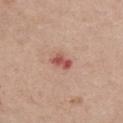| field | value |
|---|---|
| follow-up | no biopsy performed (imaged during a skin exam) |
| automated lesion analysis | an area of roughly 3.5 mm², an eccentricity of roughly 0.8, and a shape-asymmetry score of about 0.3 (0 = symmetric); an average lesion color of about L≈54 a*≈29 b*≈26 (CIELAB), roughly 12 lightness units darker than nearby skin, and a normalized lesion–skin contrast near 8.5; a border-irregularity rating of about 3/10 and internal color variation of about 3 on a 0–10 scale; an automated nevus-likeness rating near 0 out of 100 |
| image source | ~15 mm tile from a whole-body skin photo |
| tile lighting | white-light illumination |
| site | the chest |
| lesion diameter | ~2.5 mm (longest diameter) |
| subject | female, aged 63–67 |